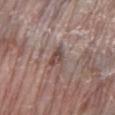workup = total-body-photography surveillance lesion; no biopsy | image source = ~15 mm tile from a whole-body skin photo | body site = the left lower leg | subject = female, aged approximately 85 or older.